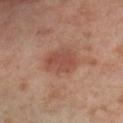- biopsy status: catalogued during a skin exam; not biopsied
- automated metrics: an average lesion color of about L≈49 a*≈24 b*≈28 (CIELAB) and a normalized border contrast of about 6.5; a border-irregularity index near 2/10, a color-variation rating of about 2.5/10, and radial color variation of about 1; a classifier nevus-likeness of about 75/100 and lesion-presence confidence of about 100/100
- illumination: cross-polarized
- anatomic site: the left thigh
- image source: ~15 mm crop, total-body skin-cancer survey
- diameter: about 4 mm
- patient: female, in their mid-50s